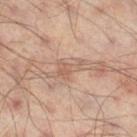Notes:
* patient — male, about 65 years old
* acquisition — ~15 mm crop, total-body skin-cancer survey
* lesion size — about 3 mm
* image-analysis metrics — an area of roughly 4.5 mm², an outline eccentricity of about 0.85 (0 = round, 1 = elongated), and a shape-asymmetry score of about 0.5 (0 = symmetric); a border-irregularity index near 6/10, a color-variation rating of about 1/10, and radial color variation of about 0.5
* illumination — cross-polarized
* site — the right thigh